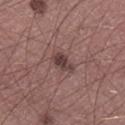Captured during whole-body skin photography for melanoma surveillance; the lesion was not biopsied. The lesion's longest dimension is about 3 mm. The lesion is located on the right lower leg. A 15 mm crop from a total-body photograph taken for skin-cancer surveillance. Captured under white-light illumination. A male subject, aged around 60.